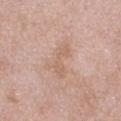Clinical impression:
Recorded during total-body skin imaging; not selected for excision or biopsy.
Acquisition and patient details:
A 15 mm crop from a total-body photograph taken for skin-cancer surveillance. Automated tile analysis of the lesion measured an area of roughly 8 mm² and two-axis asymmetry of about 0.55. It also reported border irregularity of about 7.5 on a 0–10 scale, internal color variation of about 2 on a 0–10 scale, and peripheral color asymmetry of about 0.5. And it measured an automated nevus-likeness rating near 0 out of 100 and a lesion-detection confidence of about 100/100. The recorded lesion diameter is about 4.5 mm. A male subject approximately 50 years of age. Captured under white-light illumination. The lesion is on the chest.